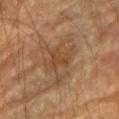Q: Was this lesion biopsied?
A: no biopsy performed (imaged during a skin exam)
Q: What lighting was used for the tile?
A: cross-polarized illumination
Q: Patient demographics?
A: male, aged approximately 85 or older
Q: Where on the body is the lesion?
A: the arm
Q: How large is the lesion?
A: ~6 mm (longest diameter)
Q: What kind of image is this?
A: ~15 mm crop, total-body skin-cancer survey